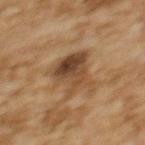The subject is a female roughly 60 years of age.
An algorithmic analysis of the crop reported a footprint of about 14 mm², an outline eccentricity of about 0.65 (0 = round, 1 = elongated), and two-axis asymmetry of about 0.4. And it measured an average lesion color of about L≈39 a*≈16 b*≈29 (CIELAB). It also reported a nevus-likeness score of about 30/100.
The lesion's longest dimension is about 5.5 mm.
A close-up tile cropped from a whole-body skin photograph, about 15 mm across.
Imaged with cross-polarized lighting.
The lesion is on the upper back.